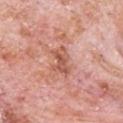Findings:
- notes — imaged on a skin check; not biopsied
- image source — 15 mm crop, total-body photography
- illumination — white-light illumination
- subject — male, about 80 years old
- site — the front of the torso
- lesion size — ≈4 mm